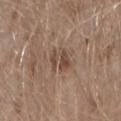Q: Was a biopsy performed?
A: imaged on a skin check; not biopsied
Q: What is the imaging modality?
A: ~15 mm crop, total-body skin-cancer survey
Q: What lighting was used for the tile?
A: white-light
Q: Automated lesion metrics?
A: a footprint of about 5 mm², an outline eccentricity of about 0.75 (0 = round, 1 = elongated), and two-axis asymmetry of about 0.35; an average lesion color of about L≈46 a*≈16 b*≈25 (CIELAB), roughly 9 lightness units darker than nearby skin, and a normalized lesion–skin contrast near 7
Q: Patient demographics?
A: male, approximately 60 years of age
Q: Lesion location?
A: the right forearm
Q: Lesion size?
A: ~3 mm (longest diameter)